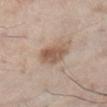follow-up: no biopsy performed (imaged during a skin exam)
anatomic site: the right lower leg
tile lighting: white-light illumination
image: 15 mm crop, total-body photography
subject: male, approximately 60 years of age